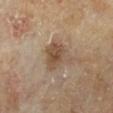The lesion was photographed on a routine skin check and not biopsied; there is no pathology result.
On the left lower leg.
A lesion tile, about 15 mm wide, cut from a 3D total-body photograph.
The patient is a male about 65 years old.
Imaged with cross-polarized lighting.
Automated image analysis of the tile measured a lesion color around L≈50 a*≈15 b*≈29 in CIELAB and a lesion–skin lightness drop of about 10.
Measured at roughly 4 mm in maximum diameter.